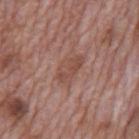{"biopsy_status": "not biopsied; imaged during a skin examination", "lighting": "white-light", "site": "mid back", "automated_metrics": {"cielab_L": 48, "cielab_a": 21, "cielab_b": 26, "vs_skin_darker_L": 7.0, "border_irregularity_0_10": 3.5, "color_variation_0_10": 3.0, "nevus_likeness_0_100": 0, "lesion_detection_confidence_0_100": 100}, "lesion_size": {"long_diameter_mm_approx": 4.0}, "image": {"source": "total-body photography crop", "field_of_view_mm": 15}, "patient": {"sex": "male", "age_approx": 70}}